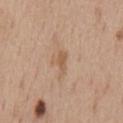follow-up = imaged on a skin check; not biopsied